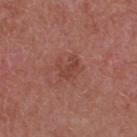The lesion was tiled from a total-body skin photograph and was not biopsied.
On the upper back.
Longest diameter approximately 3 mm.
A female patient, roughly 60 years of age.
Cropped from a total-body skin-imaging series; the visible field is about 15 mm.
The tile uses white-light illumination.